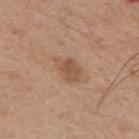Captured during whole-body skin photography for melanoma surveillance; the lesion was not biopsied.